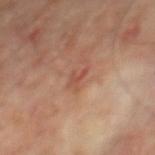{
  "biopsy_status": "not biopsied; imaged during a skin examination"
}